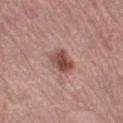workup = no biopsy performed (imaged during a skin exam).